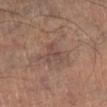Impression: This lesion was catalogued during total-body skin photography and was not selected for biopsy. Clinical summary: The recorded lesion diameter is about 3 mm. A male subject, aged 58 to 62. The tile uses cross-polarized illumination. The total-body-photography lesion software estimated a lesion area of about 4.5 mm², a shape eccentricity near 0.7, and a symmetry-axis asymmetry near 0.6. It also reported a border-irregularity index near 7.5/10, a within-lesion color-variation index near 2.5/10, and radial color variation of about 1. The lesion is located on the leg. A close-up tile cropped from a whole-body skin photograph, about 15 mm across.